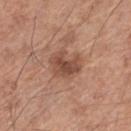• biopsy status: total-body-photography surveillance lesion; no biopsy
• size: ≈4 mm
• subject: male, about 65 years old
• body site: the leg
• image: ~15 mm crop, total-body skin-cancer survey